Measured at roughly 1 mm in maximum diameter. A male patient aged approximately 65. Imaged with white-light lighting. The lesion is on the chest. Cropped from a total-body skin-imaging series; the visible field is about 15 mm — 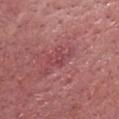Q: What did the biopsy show?
A: an actinic keratosis (borderline)